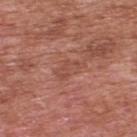notes = no biopsy performed (imaged during a skin exam).